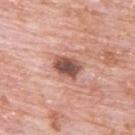Notes:
- workup · total-body-photography surveillance lesion; no biopsy
- lesion size · ~3.5 mm (longest diameter)
- imaging modality · 15 mm crop, total-body photography
- anatomic site · the upper back
- illumination · white-light illumination
- patient · male, roughly 80 years of age
- automated lesion analysis · an area of roughly 7.5 mm² and a symmetry-axis asymmetry near 0.2